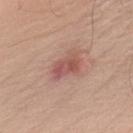Assessment: Imaged during a routine full-body skin examination; the lesion was not biopsied and no histopathology is available. Clinical summary: A lesion tile, about 15 mm wide, cut from a 3D total-body photograph. The subject is a male about 50 years old. Located on the right upper arm. The recorded lesion diameter is about 4 mm. This is a white-light tile.